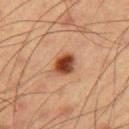Imaged during a routine full-body skin examination; the lesion was not biopsied and no histopathology is available.
The lesion is located on the right upper arm.
Imaged with cross-polarized lighting.
A male subject, approximately 55 years of age.
The lesion's longest dimension is about 3 mm.
Cropped from a whole-body photographic skin survey; the tile spans about 15 mm.